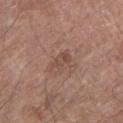workup: imaged on a skin check; not biopsied | location: the left lower leg | image source: total-body-photography crop, ~15 mm field of view | subject: male, aged 78–82.